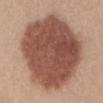notes = catalogued during a skin exam; not biopsied
body site = the abdomen
image source = ~15 mm crop, total-body skin-cancer survey
subject = female, aged 43 to 47